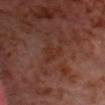Q: Was this lesion biopsied?
A: imaged on a skin check; not biopsied
Q: Lesion size?
A: ≈3 mm
Q: Automated lesion metrics?
A: an average lesion color of about L≈31 a*≈23 b*≈27 (CIELAB), about 5 CIELAB-L* units darker than the surrounding skin, and a normalized lesion–skin contrast near 5.5; a border-irregularity rating of about 5/10, internal color variation of about 1.5 on a 0–10 scale, and peripheral color asymmetry of about 0.5
Q: Patient demographics?
A: male, roughly 55 years of age
Q: Where on the body is the lesion?
A: the head or neck
Q: How was this image acquired?
A: total-body-photography crop, ~15 mm field of view
Q: What lighting was used for the tile?
A: cross-polarized